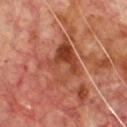workup: catalogued during a skin exam; not biopsied
lesion diameter: ≈5.5 mm
subject: male, aged approximately 70
body site: the chest
image source: 15 mm crop, total-body photography
tile lighting: cross-polarized illumination